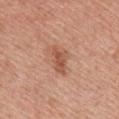About 4 mm across. The tile uses white-light illumination. A region of skin cropped from a whole-body photographic capture, roughly 15 mm wide. A female subject, in their mid- to late 50s. On the front of the torso.